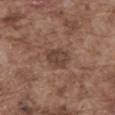Recorded during total-body skin imaging; not selected for excision or biopsy. A lesion tile, about 15 mm wide, cut from a 3D total-body photograph. The lesion-visualizer software estimated an area of roughly 7 mm² and a symmetry-axis asymmetry near 0.2. Longest diameter approximately 3.5 mm. The lesion is on the abdomen. Captured under white-light illumination. The patient is a male in their mid- to late 70s.